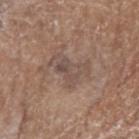The lesion was photographed on a routine skin check and not biopsied; there is no pathology result. An algorithmic analysis of the crop reported a footprint of about 9 mm², a shape eccentricity near 0.85, and a symmetry-axis asymmetry near 0.55. The software also gave a mean CIELAB color near L≈49 a*≈15 b*≈23 and about 7 CIELAB-L* units darker than the surrounding skin. The analysis additionally found border irregularity of about 6.5 on a 0–10 scale, a color-variation rating of about 4.5/10, and a peripheral color-asymmetry measure near 1.5. The lesion's longest dimension is about 5 mm. A roughly 15 mm field-of-view crop from a total-body skin photograph. From the right forearm. Imaged with white-light lighting. A female patient about 75 years old.